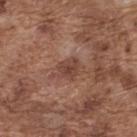Captured during whole-body skin photography for melanoma surveillance; the lesion was not biopsied.
The lesion-visualizer software estimated border irregularity of about 2.5 on a 0–10 scale and radial color variation of about 0.5. The analysis additionally found a detector confidence of about 100 out of 100 that the crop contains a lesion.
Captured under white-light illumination.
The lesion is located on the upper back.
Longest diameter approximately 3 mm.
A lesion tile, about 15 mm wide, cut from a 3D total-body photograph.
The subject is a male aged approximately 75.